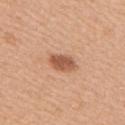workup = no biopsy performed (imaged during a skin exam)